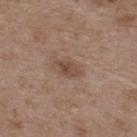Recorded during total-body skin imaging; not selected for excision or biopsy.
A male subject aged approximately 50.
A roughly 15 mm field-of-view crop from a total-body skin photograph.
On the back.
This is a white-light tile.
Approximately 3 mm at its widest.